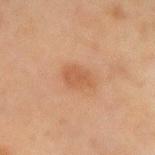No biopsy was performed on this lesion — it was imaged during a full skin examination and was not determined to be concerning. Approximately 3 mm at its widest. A male patient, aged approximately 65. On the left forearm. The lesion-visualizer software estimated a lesion color around L≈46 a*≈19 b*≈30 in CIELAB, a lesion–skin lightness drop of about 6, and a normalized lesion–skin contrast near 5.5. The analysis additionally found a border-irregularity rating of about 2/10 and a peripheral color-asymmetry measure near 0.5. A 15 mm close-up tile from a total-body photography series done for melanoma screening.